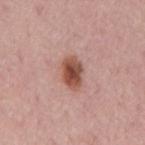workup = catalogued during a skin exam; not biopsied | imaging modality = ~15 mm tile from a whole-body skin photo | patient = male, about 75 years old | anatomic site = the mid back.